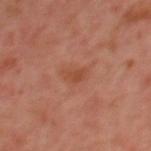Q: Was this lesion biopsied?
A: no biopsy performed (imaged during a skin exam)
Q: What is the lesion's diameter?
A: ≈2.5 mm
Q: How was the tile lit?
A: cross-polarized
Q: What did automated image analysis measure?
A: a nevus-likeness score of about 5/100 and a lesion-detection confidence of about 100/100
Q: What are the patient's age and sex?
A: male, in their mid- to late 60s
Q: How was this image acquired?
A: ~15 mm crop, total-body skin-cancer survey
Q: What is the anatomic site?
A: the upper back